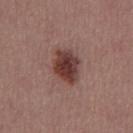The lesion was photographed on a routine skin check and not biopsied; there is no pathology result.
Cropped from a whole-body photographic skin survey; the tile spans about 15 mm.
Imaged with white-light lighting.
From the chest.
The total-body-photography lesion software estimated an eccentricity of roughly 0.65 and two-axis asymmetry of about 0.15. The software also gave an automated nevus-likeness rating near 95 out of 100 and a lesion-detection confidence of about 100/100.
A male subject, roughly 40 years of age.